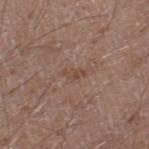No biopsy was performed on this lesion — it was imaged during a full skin examination and was not determined to be concerning. A male subject in their 20s. From the leg. Imaged with white-light lighting. A 15 mm crop from a total-body photograph taken for skin-cancer surveillance.